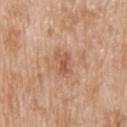Clinical impression: This lesion was catalogued during total-body skin photography and was not selected for biopsy. Context: Imaged with white-light lighting. The lesion is located on the upper back. A 15 mm crop from a total-body photograph taken for skin-cancer surveillance. The patient is a male aged approximately 80. Longest diameter approximately 3 mm.